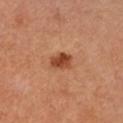This lesion was catalogued during total-body skin photography and was not selected for biopsy.
A close-up tile cropped from a whole-body skin photograph, about 15 mm across.
The lesion is on the right forearm.
Imaged with cross-polarized lighting.
A female subject aged 33–37.
About 2.5 mm across.